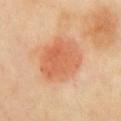The lesion was tiled from a total-body skin photograph and was not biopsied.
A female patient in their 40s.
This image is a 15 mm lesion crop taken from a total-body photograph.
The tile uses cross-polarized illumination.
Located on the chest.